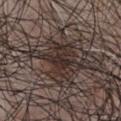The lesion was tiled from a total-body skin photograph and was not biopsied. A male patient, aged 48 to 52. From the chest. Cropped from a total-body skin-imaging series; the visible field is about 15 mm.